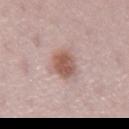No biopsy was performed on this lesion — it was imaged during a full skin examination and was not determined to be concerning.
A 15 mm crop from a total-body photograph taken for skin-cancer surveillance.
Located on the right upper arm.
A female patient aged 43–47.